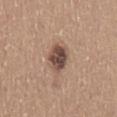workup=no biopsy performed (imaged during a skin exam) | acquisition=15 mm crop, total-body photography | tile lighting=white-light illumination | lesion size=≈3.5 mm | subject=male, in their mid- to late 60s | anatomic site=the mid back.